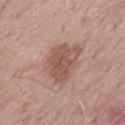TBP lesion metrics = a mean CIELAB color near L≈54 a*≈21 b*≈25 and a lesion–skin lightness drop of about 10; a color-variation rating of about 3.5/10 | anatomic site = the back | lesion size = about 6 mm | lighting = white-light | subject = male, approximately 70 years of age | image source = ~15 mm crop, total-body skin-cancer survey.